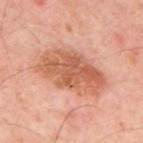Assessment: Part of a total-body skin-imaging series; this lesion was reviewed on a skin check and was not flagged for biopsy. Context: Cropped from a whole-body photographic skin survey; the tile spans about 15 mm. Imaged with cross-polarized lighting. Approximately 8 mm at its widest. The lesion is located on the mid back. The patient is aged 53 to 57. An algorithmic analysis of the crop reported a footprint of about 26 mm², an eccentricity of roughly 0.85, and two-axis asymmetry of about 0.15. It also reported a lesion color around L≈59 a*≈26 b*≈33 in CIELAB. It also reported a classifier nevus-likeness of about 0/100 and lesion-presence confidence of about 100/100.